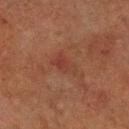Clinical impression: The lesion was tiled from a total-body skin photograph and was not biopsied. Image and clinical context: On the left lower leg. A male subject about 70 years old. Captured under cross-polarized illumination. The lesion-visualizer software estimated a border-irregularity rating of about 4/10 and internal color variation of about 4 on a 0–10 scale. The software also gave an automated nevus-likeness rating near 0 out of 100. A lesion tile, about 15 mm wide, cut from a 3D total-body photograph.